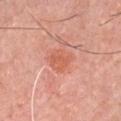Image and clinical context: The subject is a male roughly 45 years of age. The lesion is on the chest. Longest diameter approximately 3 mm. The tile uses white-light illumination. Cropped from a whole-body photographic skin survey; the tile spans about 15 mm.